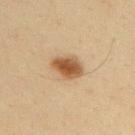illumination: cross-polarized | diameter: ~4 mm (longest diameter) | subject: male, aged approximately 35 | image-analysis metrics: an average lesion color of about L≈49 a*≈18 b*≈34 (CIELAB), about 14 CIELAB-L* units darker than the surrounding skin, and a lesion-to-skin contrast of about 10 (normalized; higher = more distinct); a border-irregularity index near 2/10 | image source: total-body-photography crop, ~15 mm field of view | site: the upper back.